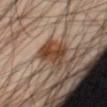Clinical impression: The lesion was photographed on a routine skin check and not biopsied; there is no pathology result. Image and clinical context: The total-body-photography lesion software estimated a footprint of about 10 mm², an eccentricity of roughly 0.6, and two-axis asymmetry of about 0.3. It also reported a classifier nevus-likeness of about 95/100 and a detector confidence of about 100 out of 100 that the crop contains a lesion. A 15 mm close-up tile from a total-body photography series done for melanoma screening. Captured under cross-polarized illumination. The lesion is on the lower back. A male subject, aged 43 to 47. The lesion's longest dimension is about 4 mm.